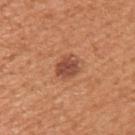Assessment: Captured during whole-body skin photography for melanoma surveillance; the lesion was not biopsied. Clinical summary: The patient is a male roughly 55 years of age. Measured at roughly 3 mm in maximum diameter. Automated image analysis of the tile measured a lesion area of about 6 mm² and two-axis asymmetry of about 0.15. And it measured a mean CIELAB color near L≈49 a*≈26 b*≈32, roughly 11 lightness units darker than nearby skin, and a normalized border contrast of about 8.5. It also reported a detector confidence of about 100 out of 100 that the crop contains a lesion. From the upper back. This is a white-light tile. A lesion tile, about 15 mm wide, cut from a 3D total-body photograph.